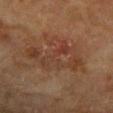Q: Was a biopsy performed?
A: imaged on a skin check; not biopsied
Q: Lesion size?
A: ≈7.5 mm
Q: What did automated image analysis measure?
A: a lesion color around L≈33 a*≈18 b*≈25 in CIELAB and about 5 CIELAB-L* units darker than the surrounding skin; an automated nevus-likeness rating near 0 out of 100
Q: Patient demographics?
A: female, aged 78–82
Q: How was this image acquired?
A: total-body-photography crop, ~15 mm field of view
Q: What is the anatomic site?
A: the right forearm